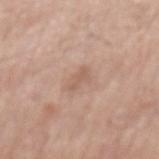Assessment: No biopsy was performed on this lesion — it was imaged during a full skin examination and was not determined to be concerning. Acquisition and patient details: Automated image analysis of the tile measured border irregularity of about 3.5 on a 0–10 scale, a color-variation rating of about 0/10, and a peripheral color-asymmetry measure near 0. It also reported a nevus-likeness score of about 0/100 and a detector confidence of about 100 out of 100 that the crop contains a lesion. Measured at roughly 2.5 mm in maximum diameter. From the mid back. A male patient aged around 80. A 15 mm close-up extracted from a 3D total-body photography capture. Captured under white-light illumination.